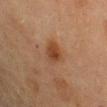Q: Is there a histopathology result?
A: imaged on a skin check; not biopsied
Q: What is the lesion's diameter?
A: about 3.5 mm
Q: How was this image acquired?
A: ~15 mm tile from a whole-body skin photo
Q: Where on the body is the lesion?
A: the left upper arm
Q: Who is the patient?
A: female, about 70 years old
Q: What lighting was used for the tile?
A: cross-polarized illumination